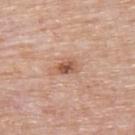{"biopsy_status": "not biopsied; imaged during a skin examination", "lesion_size": {"long_diameter_mm_approx": 3.0}, "lighting": "white-light", "site": "upper back", "image": {"source": "total-body photography crop", "field_of_view_mm": 15}, "patient": {"sex": "male", "age_approx": 75}, "automated_metrics": {"cielab_L": 56, "cielab_a": 23, "cielab_b": 31, "vs_skin_darker_L": 12.0, "vs_skin_contrast_norm": 8.0}}